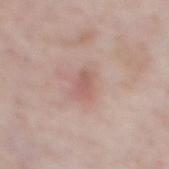Case summary:
* subject · male, aged around 60
* image source · ~15 mm crop, total-body skin-cancer survey
* location · the mid back